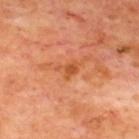Assessment:
This lesion was catalogued during total-body skin photography and was not selected for biopsy.
Image and clinical context:
This image is a 15 mm lesion crop taken from a total-body photograph. Located on the back. A patient in their mid-60s. This is a cross-polarized tile. The total-body-photography lesion software estimated a border-irregularity rating of about 5.5/10, internal color variation of about 1.5 on a 0–10 scale, and radial color variation of about 0.5. Measured at roughly 2.5 mm in maximum diameter.